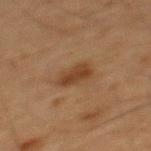Impression:
The lesion was photographed on a routine skin check and not biopsied; there is no pathology result.
Acquisition and patient details:
Measured at roughly 3 mm in maximum diameter. The tile uses cross-polarized illumination. The lesion is located on the mid back. An algorithmic analysis of the crop reported an eccentricity of roughly 0.8 and a symmetry-axis asymmetry near 0.2. The analysis additionally found an automated nevus-likeness rating near 80 out of 100. A roughly 15 mm field-of-view crop from a total-body skin photograph. A male patient roughly 65 years of age.